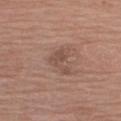follow-up: no biopsy performed (imaged during a skin exam)
location: the right upper arm
image: ~15 mm crop, total-body skin-cancer survey
lighting: white-light
subject: male, roughly 60 years of age